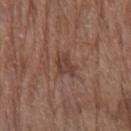workup — total-body-photography surveillance lesion; no biopsy
location — the left forearm
imaging modality — ~15 mm crop, total-body skin-cancer survey
illumination — white-light
subject — female, about 80 years old